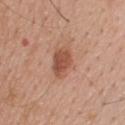Q: Was a biopsy performed?
A: catalogued during a skin exam; not biopsied